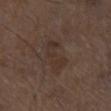Assessment:
Imaged during a routine full-body skin examination; the lesion was not biopsied and no histopathology is available.
Acquisition and patient details:
The recorded lesion diameter is about 4.5 mm. A male patient, in their mid- to late 70s. Cropped from a whole-body photographic skin survey; the tile spans about 15 mm. From the right thigh. Captured under white-light illumination. Automated tile analysis of the lesion measured a footprint of about 7.5 mm² and a shape eccentricity near 0.9. The analysis additionally found a mean CIELAB color near L≈31 a*≈14 b*≈21 and a normalized lesion–skin contrast near 5.5. It also reported internal color variation of about 2 on a 0–10 scale and peripheral color asymmetry of about 0.5. The analysis additionally found an automated nevus-likeness rating near 0 out of 100.